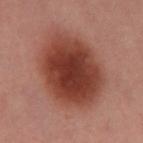Clinical summary:
From the left thigh. A female subject, approximately 40 years of age. This image is a 15 mm lesion crop taken from a total-body photograph. The recorded lesion diameter is about 9 mm. Automated image analysis of the tile measured an average lesion color of about L≈40 a*≈27 b*≈28 (CIELAB), a lesion–skin lightness drop of about 15, and a normalized border contrast of about 11.5.